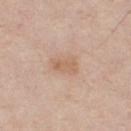Assessment:
Part of a total-body skin-imaging series; this lesion was reviewed on a skin check and was not flagged for biopsy.
Clinical summary:
The recorded lesion diameter is about 3 mm. A male patient, aged 43–47. The lesion is located on the chest. Imaged with white-light lighting. A 15 mm crop from a total-body photograph taken for skin-cancer surveillance. The total-body-photography lesion software estimated a lesion area of about 5 mm², a shape eccentricity near 0.8, and two-axis asymmetry of about 0.3. And it measured a lesion–skin lightness drop of about 7 and a lesion-to-skin contrast of about 6 (normalized; higher = more distinct). It also reported border irregularity of about 3 on a 0–10 scale, a within-lesion color-variation index near 2/10, and peripheral color asymmetry of about 0.5. The software also gave lesion-presence confidence of about 100/100.